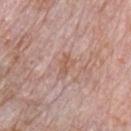The lesion was tiled from a total-body skin photograph and was not biopsied.
Imaged with white-light lighting.
From the chest.
The lesion's longest dimension is about 3 mm.
An algorithmic analysis of the crop reported a mean CIELAB color near L≈57 a*≈20 b*≈27 and a normalized border contrast of about 5.5.
Cropped from a whole-body photographic skin survey; the tile spans about 15 mm.
The subject is a male about 70 years old.